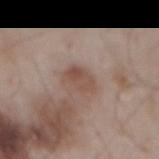biopsy_status: not biopsied; imaged during a skin examination
lighting: white-light
automated_metrics:
  eccentricity: 0.7
  shape_asymmetry: 0.2
  vs_skin_darker_L: 8.0
  vs_skin_contrast_norm: 6.5
  border_irregularity_0_10: 2.0
  color_variation_0_10: 4.0
  peripheral_color_asymmetry: 1.5
site: mid back
patient:
  sex: male
  age_approx: 55
image:
  source: total-body photography crop
  field_of_view_mm: 15